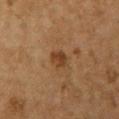Part of a total-body skin-imaging series; this lesion was reviewed on a skin check and was not flagged for biopsy.
The lesion's longest dimension is about 2.5 mm.
This image is a 15 mm lesion crop taken from a total-body photograph.
Captured under cross-polarized illumination.
The lesion is located on the front of the torso.
A female patient aged 58 to 62.